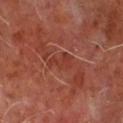| key | value |
|---|---|
| workup | total-body-photography surveillance lesion; no biopsy |
| patient | male, approximately 65 years of age |
| lighting | cross-polarized |
| anatomic site | the chest |
| acquisition | ~15 mm crop, total-body skin-cancer survey |
| lesion diameter | ~3 mm (longest diameter) |